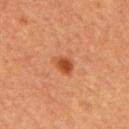biopsy status=no biopsy performed (imaged during a skin exam) | subject=male, aged 63–67 | image source=~15 mm crop, total-body skin-cancer survey | site=the chest.